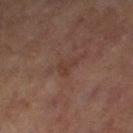follow-up = imaged on a skin check; not biopsied | location = the left thigh | automated lesion analysis = a mean CIELAB color near L≈32 a*≈17 b*≈21, a lesion–skin lightness drop of about 5, and a normalized border contrast of about 5; a border-irregularity rating of about 4/10, internal color variation of about 1.5 on a 0–10 scale, and peripheral color asymmetry of about 0.5; a nevus-likeness score of about 0/100 and a detector confidence of about 100 out of 100 that the crop contains a lesion | imaging modality = total-body-photography crop, ~15 mm field of view | patient = female, about 60 years old | lighting = cross-polarized.